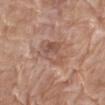Assessment: Imaged during a routine full-body skin examination; the lesion was not biopsied and no histopathology is available. Acquisition and patient details: A region of skin cropped from a whole-body photographic capture, roughly 15 mm wide. A female subject, aged around 75. Located on the right thigh.